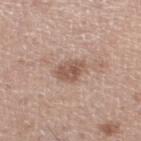Impression:
Captured during whole-body skin photography for melanoma surveillance; the lesion was not biopsied.
Context:
A region of skin cropped from a whole-body photographic capture, roughly 15 mm wide. A male subject aged 63–67. The lesion-visualizer software estimated a border-irregularity index near 2/10, a within-lesion color-variation index near 3/10, and a peripheral color-asymmetry measure near 1. The lesion is located on the left lower leg.